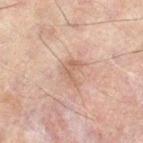{
  "biopsy_status": "not biopsied; imaged during a skin examination",
  "site": "left thigh",
  "patient": {
    "sex": "male",
    "age_approx": 60
  },
  "lesion_size": {
    "long_diameter_mm_approx": 3.0
  },
  "automated_metrics": {
    "area_mm2_approx": 4.5,
    "eccentricity": 0.75,
    "shape_asymmetry": 0.55,
    "vs_skin_contrast_norm": 5.5,
    "color_variation_0_10": 1.5,
    "peripheral_color_asymmetry": 0.5,
    "nevus_likeness_0_100": 0,
    "lesion_detection_confidence_0_100": 100
  },
  "lighting": "cross-polarized",
  "image": {
    "source": "total-body photography crop",
    "field_of_view_mm": 15
  }
}